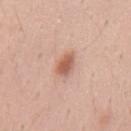| field | value |
|---|---|
| follow-up | catalogued during a skin exam; not biopsied |
| diameter | ~3 mm (longest diameter) |
| lighting | white-light illumination |
| patient | male, aged around 40 |
| acquisition | 15 mm crop, total-body photography |
| image-analysis metrics | a mean CIELAB color near L≈59 a*≈23 b*≈30, a lesion–skin lightness drop of about 12, and a normalized border contrast of about 8 |
| body site | the mid back |